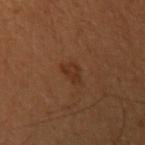Q: Was this lesion biopsied?
A: imaged on a skin check; not biopsied
Q: How was the tile lit?
A: cross-polarized
Q: Where on the body is the lesion?
A: the arm
Q: Automated lesion metrics?
A: a lesion area of about 3.5 mm², an outline eccentricity of about 0.7 (0 = round, 1 = elongated), and two-axis asymmetry of about 0.4; border irregularity of about 4 on a 0–10 scale, a within-lesion color-variation index near 1.5/10, and radial color variation of about 0.5
Q: What is the lesion's diameter?
A: ≈2.5 mm
Q: What kind of image is this?
A: ~15 mm crop, total-body skin-cancer survey
Q: Who is the patient?
A: female, roughly 50 years of age